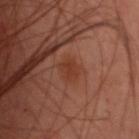Captured during whole-body skin photography for melanoma surveillance; the lesion was not biopsied. A 15 mm crop from a total-body photograph taken for skin-cancer surveillance. Imaged with cross-polarized lighting. The lesion's longest dimension is about 2.5 mm. An algorithmic analysis of the crop reported a mean CIELAB color near L≈37 a*≈24 b*≈30, about 5 CIELAB-L* units darker than the surrounding skin, and a normalized border contrast of about 6. The analysis additionally found a border-irregularity rating of about 1.5/10 and a color-variation rating of about 1.5/10. It also reported a lesion-detection confidence of about 100/100. A female patient, approximately 40 years of age. On the upper back.